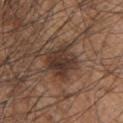Automated tile analysis of the lesion measured an area of roughly 12 mm² and a shape-asymmetry score of about 0.25 (0 = symmetric). The software also gave a mean CIELAB color near L≈35 a*≈17 b*≈23, about 11 CIELAB-L* units darker than the surrounding skin, and a normalized lesion–skin contrast near 10. Located on the upper back. Cropped from a total-body skin-imaging series; the visible field is about 15 mm. A male patient, approximately 45 years of age.